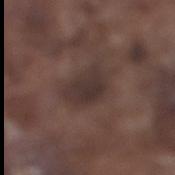No biopsy was performed on this lesion — it was imaged during a full skin examination and was not determined to be concerning.
Approximately 4.5 mm at its widest.
The subject is a male roughly 75 years of age.
A region of skin cropped from a whole-body photographic capture, roughly 15 mm wide.
Automated tile analysis of the lesion measured a lesion area of about 9 mm², an eccentricity of roughly 0.8, and a symmetry-axis asymmetry near 0.25. The analysis additionally found a border-irregularity rating of about 2.5/10, a within-lesion color-variation index near 2.5/10, and peripheral color asymmetry of about 1. The analysis additionally found lesion-presence confidence of about 85/100.
On the right lower leg.
This is a white-light tile.